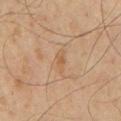{"biopsy_status": "not biopsied; imaged during a skin examination", "patient": {"sex": "male", "age_approx": 60}, "site": "chest", "image": {"source": "total-body photography crop", "field_of_view_mm": 15}, "lesion_size": {"long_diameter_mm_approx": 3.0}}